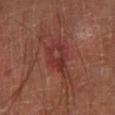Approximately 4.5 mm at its widest. A 15 mm close-up extracted from a 3D total-body photography capture. Automated image analysis of the tile measured an area of roughly 8.5 mm². The analysis additionally found a classifier nevus-likeness of about 0/100 and a detector confidence of about 75 out of 100 that the crop contains a lesion. Imaged with cross-polarized lighting. A male patient, about 65 years old. The lesion is located on the left lower leg.